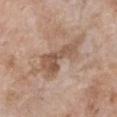Part of a total-body skin-imaging series; this lesion was reviewed on a skin check and was not flagged for biopsy. A female patient, about 75 years old. A close-up tile cropped from a whole-body skin photograph, about 15 mm across. On the chest. Measured at roughly 8 mm in maximum diameter. Automated image analysis of the tile measured a border-irregularity rating of about 7/10, a within-lesion color-variation index near 4/10, and peripheral color asymmetry of about 1. It also reported a classifier nevus-likeness of about 0/100 and a detector confidence of about 100 out of 100 that the crop contains a lesion. Imaged with white-light lighting.